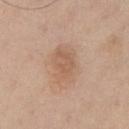Impression:
The lesion was tiled from a total-body skin photograph and was not biopsied.
Context:
Imaged with white-light lighting. The patient is a male in their 60s. On the chest. Measured at roughly 5 mm in maximum diameter. A roughly 15 mm field-of-view crop from a total-body skin photograph.